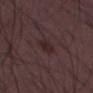Assessment:
Recorded during total-body skin imaging; not selected for excision or biopsy.
Acquisition and patient details:
From the left thigh. This image is a 15 mm lesion crop taken from a total-body photograph. The total-body-photography lesion software estimated an average lesion color of about L≈24 a*≈16 b*≈15 (CIELAB), a lesion–skin lightness drop of about 6, and a lesion-to-skin contrast of about 7 (normalized; higher = more distinct). It also reported a lesion-detection confidence of about 100/100. A male subject aged approximately 50. The recorded lesion diameter is about 3.5 mm. Imaged with white-light lighting.